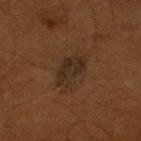Automated tile analysis of the lesion measured a lesion-detection confidence of about 95/100. The lesion is on the leg. A male patient, aged approximately 60. A roughly 15 mm field-of-view crop from a total-body skin photograph. This is a cross-polarized tile. The lesion's longest dimension is about 5 mm.